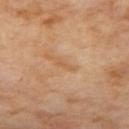Acquisition and patient details:
From the back. Cropped from a whole-body photographic skin survey; the tile spans about 15 mm. Approximately 3 mm at its widest. This is a cross-polarized tile. Automated image analysis of the tile measured a shape eccentricity near 0.95 and a shape-asymmetry score of about 0.35 (0 = symmetric). The analysis additionally found a border-irregularity index near 4/10, a within-lesion color-variation index near 0/10, and peripheral color asymmetry of about 0.